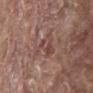  biopsy_status: not biopsied; imaged during a skin examination
  lighting: white-light
  site: back
  image:
    source: total-body photography crop
    field_of_view_mm: 15
  patient:
    sex: male
    age_approx: 80